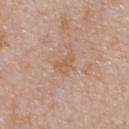{
  "biopsy_status": "not biopsied; imaged during a skin examination",
  "patient": {
    "sex": "female",
    "age_approx": 45
  },
  "site": "upper back",
  "lesion_size": {
    "long_diameter_mm_approx": 2.5
  },
  "lighting": "white-light",
  "image": {
    "source": "total-body photography crop",
    "field_of_view_mm": 15
  }
}